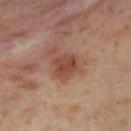Imaged during a routine full-body skin examination; the lesion was not biopsied and no histopathology is available.
About 2.5 mm across.
Automated image analysis of the tile measured a lesion color around L≈46 a*≈25 b*≈29 in CIELAB, roughly 10 lightness units darker than nearby skin, and a normalized border contrast of about 8.
A roughly 15 mm field-of-view crop from a total-body skin photograph.
A female patient roughly 55 years of age.
On the leg.
This is a cross-polarized tile.